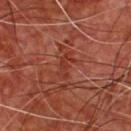Impression:
The lesion was tiled from a total-body skin photograph and was not biopsied.